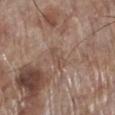lesion diameter: ~3 mm (longest diameter) | patient: male, aged 68–72 | imaging modality: 15 mm crop, total-body photography | location: the left lower leg | TBP lesion metrics: an area of roughly 3 mm², an outline eccentricity of about 0.9 (0 = round, 1 = elongated), and two-axis asymmetry of about 0.45.